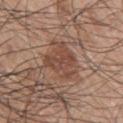Recorded during total-body skin imaging; not selected for excision or biopsy. A close-up tile cropped from a whole-body skin photograph, about 15 mm across. Longest diameter approximately 5 mm. Captured under white-light illumination. Automated tile analysis of the lesion measured a mean CIELAB color near L≈46 a*≈20 b*≈27, roughly 8 lightness units darker than nearby skin, and a normalized border contrast of about 6.5. A male patient, aged around 70. From the left arm.